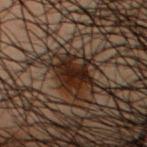Imaged during a routine full-body skin examination; the lesion was not biopsied and no histopathology is available. Imaged with cross-polarized lighting. Cropped from a total-body skin-imaging series; the visible field is about 15 mm. From the chest. Approximately 4 mm at its widest. A male subject, in their 50s.